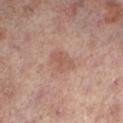No biopsy was performed on this lesion — it was imaged during a full skin examination and was not determined to be concerning. Cropped from a total-body skin-imaging series; the visible field is about 15 mm. A male patient, aged approximately 65. Imaged with cross-polarized lighting. The lesion-visualizer software estimated a lesion color around L≈52 a*≈20 b*≈25 in CIELAB, a lesion–skin lightness drop of about 7, and a normalized border contrast of about 5.5. The software also gave a border-irregularity rating of about 2.5/10, a color-variation rating of about 3/10, and a peripheral color-asymmetry measure near 1. The lesion is on the left lower leg.